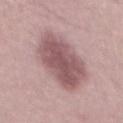  biopsy_status: not biopsied; imaged during a skin examination
  lesion_size:
    long_diameter_mm_approx: 9.0
  image:
    source: total-body photography crop
    field_of_view_mm: 15
  automated_metrics:
    vs_skin_darker_L: 14.0
  patient:
    sex: male
    age_approx: 30